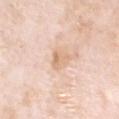Assessment: No biopsy was performed on this lesion — it was imaged during a full skin examination and was not determined to be concerning. Background: A region of skin cropped from a whole-body photographic capture, roughly 15 mm wide. On the head or neck. This is a white-light tile. Longest diameter approximately 3 mm. A male subject, aged around 80.